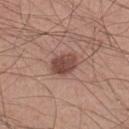| key | value |
|---|---|
| follow-up | imaged on a skin check; not biopsied |
| location | the left lower leg |
| subject | male, about 30 years old |
| imaging modality | ~15 mm tile from a whole-body skin photo |